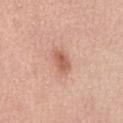{"biopsy_status": "not biopsied; imaged during a skin examination", "lighting": "white-light", "automated_metrics": {"area_mm2_approx": 5.5, "shape_asymmetry": 0.2, "border_irregularity_0_10": 2.0, "peripheral_color_asymmetry": 1.0, "lesion_detection_confidence_0_100": 100}, "site": "abdomen", "patient": {"sex": "male", "age_approx": 70}, "image": {"source": "total-body photography crop", "field_of_view_mm": 15}}